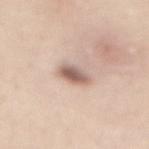Q: Is there a histopathology result?
A: catalogued during a skin exam; not biopsied
Q: What kind of image is this?
A: 15 mm crop, total-body photography
Q: Who is the patient?
A: female, in their mid- to late 20s
Q: Lesion location?
A: the chest